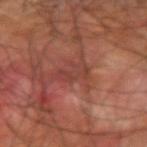Q: Was a biopsy performed?
A: imaged on a skin check; not biopsied
Q: Where on the body is the lesion?
A: the right upper arm
Q: What is the lesion's diameter?
A: about 3.5 mm
Q: What is the imaging modality?
A: total-body-photography crop, ~15 mm field of view
Q: Patient demographics?
A: male, aged around 60